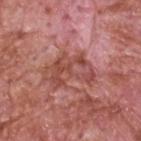Captured during whole-body skin photography for melanoma surveillance; the lesion was not biopsied. This is a white-light tile. About 5 mm across. A 15 mm close-up extracted from a 3D total-body photography capture. Automated image analysis of the tile measured an area of roughly 12 mm² and a shape-asymmetry score of about 0.45 (0 = symmetric). And it measured a border-irregularity index near 5.5/10 and internal color variation of about 7 on a 0–10 scale. A male patient roughly 60 years of age. Located on the head or neck.